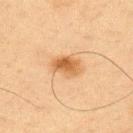image source — ~15 mm crop, total-body skin-cancer survey
size — about 3.5 mm
site — the front of the torso
illumination — cross-polarized illumination
image-analysis metrics — an area of roughly 6.5 mm² and a symmetry-axis asymmetry near 0.2; a lesion color around L≈49 a*≈19 b*≈36 in CIELAB and roughly 10 lightness units darker than nearby skin
patient — male, aged around 65